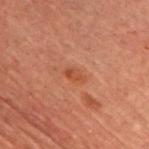| feature | finding |
|---|---|
| anatomic site | the chest |
| patient | male, roughly 60 years of age |
| image | 15 mm crop, total-body photography |
| illumination | cross-polarized |
| lesion size | ≈2.5 mm |